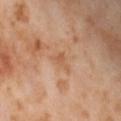biopsy status: imaged on a skin check; not biopsied
patient: female, aged 53 to 57
image: total-body-photography crop, ~15 mm field of view
location: the front of the torso
lesion diameter: ~2.5 mm (longest diameter)